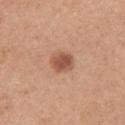Assessment:
Part of a total-body skin-imaging series; this lesion was reviewed on a skin check and was not flagged for biopsy.
Acquisition and patient details:
Located on the right upper arm. A female patient aged approximately 65. Captured under white-light illumination. Approximately 3 mm at its widest. Cropped from a whole-body photographic skin survey; the tile spans about 15 mm.